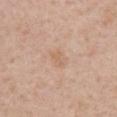Part of a total-body skin-imaging series; this lesion was reviewed on a skin check and was not flagged for biopsy. A female subject, in their 60s. The tile uses white-light illumination. The lesion is on the chest. A region of skin cropped from a whole-body photographic capture, roughly 15 mm wide. Automated tile analysis of the lesion measured a border-irregularity index near 2/10, internal color variation of about 1.5 on a 0–10 scale, and a peripheral color-asymmetry measure near 0.5. It also reported a lesion-detection confidence of about 100/100.